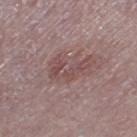No biopsy was performed on this lesion — it was imaged during a full skin examination and was not determined to be concerning.
About 4.5 mm across.
The lesion-visualizer software estimated an area of roughly 8.5 mm², an eccentricity of roughly 0.85, and two-axis asymmetry of about 0.55. And it measured a border-irregularity rating of about 7.5/10, internal color variation of about 2.5 on a 0–10 scale, and radial color variation of about 1. The analysis additionally found a lesion-detection confidence of about 100/100.
Cropped from a whole-body photographic skin survey; the tile spans about 15 mm.
The subject is a male aged 73–77.
On the left thigh.